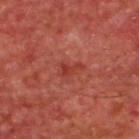Impression: The lesion was photographed on a routine skin check and not biopsied; there is no pathology result. Image and clinical context: The total-body-photography lesion software estimated a footprint of about 3.5 mm² and a shape eccentricity near 0.85. The analysis additionally found a mean CIELAB color near L≈36 a*≈31 b*≈28, about 6 CIELAB-L* units darker than the surrounding skin, and a lesion-to-skin contrast of about 5.5 (normalized; higher = more distinct). The software also gave border irregularity of about 5.5 on a 0–10 scale, a within-lesion color-variation index near 1/10, and peripheral color asymmetry of about 0. It also reported a nevus-likeness score of about 0/100 and a detector confidence of about 100 out of 100 that the crop contains a lesion. A male patient aged around 65. Longest diameter approximately 3 mm. Cropped from a total-body skin-imaging series; the visible field is about 15 mm. On the upper back.